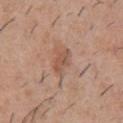{"biopsy_status": "not biopsied; imaged during a skin examination", "patient": {"sex": "male", "age_approx": 30}, "automated_metrics": {"nevus_likeness_0_100": 0, "lesion_detection_confidence_0_100": 100}, "site": "chest", "image": {"source": "total-body photography crop", "field_of_view_mm": 15}}